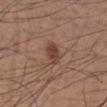From the right lower leg.
The patient is a male aged around 35.
A lesion tile, about 15 mm wide, cut from a 3D total-body photograph.
The recorded lesion diameter is about 3 mm.
An algorithmic analysis of the crop reported an average lesion color of about L≈42 a*≈20 b*≈25 (CIELAB), a lesion–skin lightness drop of about 10, and a normalized border contrast of about 8. The analysis additionally found an automated nevus-likeness rating near 90 out of 100 and a detector confidence of about 100 out of 100 that the crop contains a lesion.